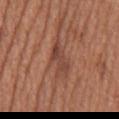Clinical impression: Recorded during total-body skin imaging; not selected for excision or biopsy. Background: On the mid back. A male patient, aged 63 to 67. A region of skin cropped from a whole-body photographic capture, roughly 15 mm wide. Measured at roughly 3.5 mm in maximum diameter. Captured under white-light illumination.